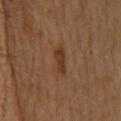| feature | finding |
|---|---|
| notes | imaged on a skin check; not biopsied |
| acquisition | 15 mm crop, total-body photography |
| location | the head or neck |
| automated metrics | an area of roughly 4.5 mm², an eccentricity of roughly 0.95, and a shape-asymmetry score of about 0.25 (0 = symmetric); border irregularity of about 3.5 on a 0–10 scale, a color-variation rating of about 1.5/10, and a peripheral color-asymmetry measure near 0.5; an automated nevus-likeness rating near 90 out of 100 and a lesion-detection confidence of about 100/100 |
| subject | male, in their mid- to late 80s or older |
| illumination | cross-polarized illumination |